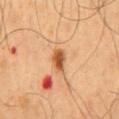Assessment: The lesion was photographed on a routine skin check and not biopsied; there is no pathology result. Context: The lesion is on the back. Captured under cross-polarized illumination. A 15 mm crop from a total-body photograph taken for skin-cancer surveillance. A male patient, aged 43–47. Approximately 3 mm at its widest.